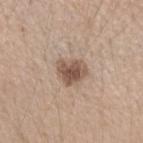{"biopsy_status": "not biopsied; imaged during a skin examination", "site": "left forearm", "patient": {"sex": "female", "age_approx": 45}, "automated_metrics": {"area_mm2_approx": 8.0, "shape_asymmetry": 0.25, "vs_skin_darker_L": 13.0}, "lesion_size": {"long_diameter_mm_approx": 3.5}, "image": {"source": "total-body photography crop", "field_of_view_mm": 15}}